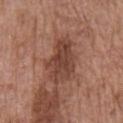Background:
Located on the chest. Automated tile analysis of the lesion measured a footprint of about 14 mm² and a shape eccentricity near 0.85. And it measured an average lesion color of about L≈42 a*≈22 b*≈27 (CIELAB), about 11 CIELAB-L* units darker than the surrounding skin, and a lesion-to-skin contrast of about 8.5 (normalized; higher = more distinct). The software also gave a border-irregularity rating of about 3.5/10 and peripheral color asymmetry of about 1. It also reported an automated nevus-likeness rating near 30 out of 100 and lesion-presence confidence of about 100/100. A 15 mm close-up extracted from a 3D total-body photography capture. The subject is a male in their mid- to late 70s. The tile uses white-light illumination.